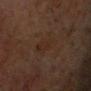Assessment:
The lesion was photographed on a routine skin check and not biopsied; there is no pathology result.
Acquisition and patient details:
From the head or neck. A male patient aged 63–67. A 15 mm close-up tile from a total-body photography series done for melanoma screening.